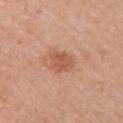follow-up: imaged on a skin check; not biopsied | subject: female, in their mid- to late 50s | image: total-body-photography crop, ~15 mm field of view | lesion diameter: ~3.5 mm (longest diameter) | anatomic site: the left upper arm.